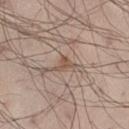follow-up: imaged on a skin check; not biopsied | subject: male, aged 28 to 32 | automated metrics: a within-lesion color-variation index near 1/10 and peripheral color asymmetry of about 0.5 | size: ~2.5 mm (longest diameter) | imaging modality: 15 mm crop, total-body photography | location: the leg.